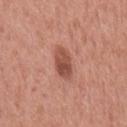Notes:
• follow-up · imaged on a skin check; not biopsied
• TBP lesion metrics · an area of roughly 7 mm², an outline eccentricity of about 0.85 (0 = round, 1 = elongated), and a shape-asymmetry score of about 0.2 (0 = symmetric); a lesion-to-skin contrast of about 8 (normalized; higher = more distinct); border irregularity of about 2 on a 0–10 scale, a color-variation rating of about 5/10, and radial color variation of about 2; a nevus-likeness score of about 80/100 and a lesion-detection confidence of about 100/100
• acquisition · ~15 mm tile from a whole-body skin photo
• body site · the mid back
• patient · male, approximately 55 years of age
• size · ≈4 mm
• illumination · white-light illumination